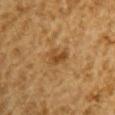Part of a total-body skin-imaging series; this lesion was reviewed on a skin check and was not flagged for biopsy. Approximately 2.5 mm at its widest. A male patient, aged approximately 85 or older. This is a cross-polarized tile. On the arm. Cropped from a total-body skin-imaging series; the visible field is about 15 mm.